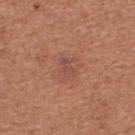• follow-up · total-body-photography surveillance lesion; no biopsy
• lighting · white-light
• subject · female, aged around 50
• image · ~15 mm crop, total-body skin-cancer survey
• site · the back